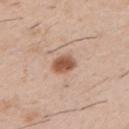No biopsy was performed on this lesion — it was imaged during a full skin examination and was not determined to be concerning.
Approximately 2.5 mm at its widest.
From the left upper arm.
A male patient aged 48 to 52.
A 15 mm crop from a total-body photograph taken for skin-cancer surveillance.
Captured under white-light illumination.